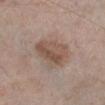No biopsy was performed on this lesion — it was imaged during a full skin examination and was not determined to be concerning. The tile uses white-light illumination. Cropped from a whole-body photographic skin survey; the tile spans about 15 mm. Approximately 5 mm at its widest. On the right lower leg. A male patient, approximately 60 years of age.